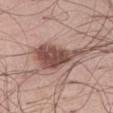The lesion was tiled from a total-body skin photograph and was not biopsied.
Measured at roughly 7.5 mm in maximum diameter.
A 15 mm crop from a total-body photograph taken for skin-cancer surveillance.
A male subject aged 18 to 22.
The lesion is on the right thigh.
The total-body-photography lesion software estimated an area of roughly 15 mm² and a symmetry-axis asymmetry near 0.5. It also reported a border-irregularity rating of about 6/10, internal color variation of about 4 on a 0–10 scale, and a peripheral color-asymmetry measure near 1.5.